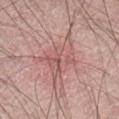Q: What kind of image is this?
A: 15 mm crop, total-body photography
Q: Patient demographics?
A: male, roughly 65 years of age
Q: Lesion location?
A: the back